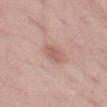workup: imaged on a skin check; not biopsied
imaging modality: ~15 mm crop, total-body skin-cancer survey
subject: male, aged around 50
lighting: white-light
size: about 2.5 mm
automated metrics: a classifier nevus-likeness of about 55/100 and a lesion-detection confidence of about 100/100
location: the left thigh